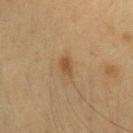| key | value |
|---|---|
| biopsy status | no biopsy performed (imaged during a skin exam) |
| image-analysis metrics | a lesion area of about 3 mm², an eccentricity of roughly 0.85, and two-axis asymmetry of about 0.3; roughly 8 lightness units darker than nearby skin and a normalized border contrast of about 7; an automated nevus-likeness rating near 60 out of 100 and a lesion-detection confidence of about 100/100 |
| image | 15 mm crop, total-body photography |
| location | the left forearm |
| patient | female, aged around 40 |
| illumination | cross-polarized |